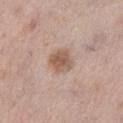Impression:
This lesion was catalogued during total-body skin photography and was not selected for biopsy.
Image and clinical context:
A female patient, aged approximately 65. The lesion is on the left lower leg. A 15 mm close-up tile from a total-body photography series done for melanoma screening.